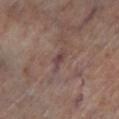Notes:
• body site · the left lower leg
• illumination · cross-polarized
• diameter · ≈3 mm
• image · total-body-photography crop, ~15 mm field of view